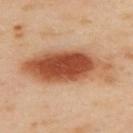{"biopsy_status": "not biopsied; imaged during a skin examination", "lesion_size": {"long_diameter_mm_approx": 10.5}, "image": {"source": "total-body photography crop", "field_of_view_mm": 15}, "site": "upper back", "lighting": "cross-polarized", "patient": {"sex": "female", "age_approx": 40}}